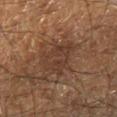Located on the right lower leg. A male subject aged approximately 60. This image is a 15 mm lesion crop taken from a total-body photograph.